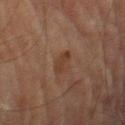| key | value |
|---|---|
| workup | no biopsy performed (imaged during a skin exam) |
| site | the right thigh |
| patient | male, aged 83 or older |
| imaging modality | ~15 mm tile from a whole-body skin photo |